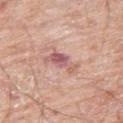Cropped from a total-body skin-imaging series; the visible field is about 15 mm. On the right thigh. The lesion's longest dimension is about 4 mm. The subject is a male aged 78–82.